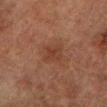{
  "biopsy_status": "not biopsied; imaged during a skin examination",
  "image": {
    "source": "total-body photography crop",
    "field_of_view_mm": 15
  },
  "automated_metrics": {
    "vs_skin_darker_L": 5.0,
    "vs_skin_contrast_norm": 5.5
  },
  "lighting": "cross-polarized",
  "site": "left lower leg",
  "patient": {
    "sex": "male",
    "age_approx": 75
  },
  "lesion_size": {
    "long_diameter_mm_approx": 2.5
  }
}